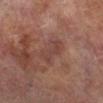Q: Was this lesion biopsied?
A: total-body-photography surveillance lesion; no biopsy
Q: What kind of image is this?
A: ~15 mm crop, total-body skin-cancer survey
Q: What is the anatomic site?
A: the leg
Q: What did automated image analysis measure?
A: a border-irregularity index near 5.5/10 and internal color variation of about 1 on a 0–10 scale; a nevus-likeness score of about 0/100 and a lesion-detection confidence of about 100/100
Q: What are the patient's age and sex?
A: male, about 75 years old
Q: How large is the lesion?
A: about 4 mm